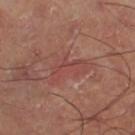| feature | finding |
|---|---|
| workup | catalogued during a skin exam; not biopsied |
| site | the right thigh |
| lesion size | ≈4.5 mm |
| acquisition | ~15 mm tile from a whole-body skin photo |